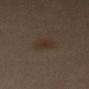biopsy status: total-body-photography surveillance lesion; no biopsy | illumination: cross-polarized illumination | lesion diameter: ~4 mm (longest diameter) | image: 15 mm crop, total-body photography | subject: female, aged 63–67 | anatomic site: the abdomen.